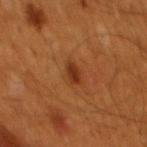biopsy status=catalogued during a skin exam; not biopsied | size=~2.5 mm (longest diameter) | site=the back | illumination=cross-polarized illumination | patient=male, aged 58 to 62 | imaging modality=15 mm crop, total-body photography.